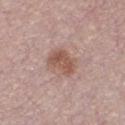The lesion was tiled from a total-body skin photograph and was not biopsied. A male patient, about 30 years old. Automated image analysis of the tile measured a lesion color around L≈54 a*≈20 b*≈26 in CIELAB and a lesion–skin lightness drop of about 10. The analysis additionally found a border-irregularity index near 3/10, a color-variation rating of about 3.5/10, and radial color variation of about 1.5. The recorded lesion diameter is about 3.5 mm. Cropped from a whole-body photographic skin survey; the tile spans about 15 mm. This is a white-light tile.